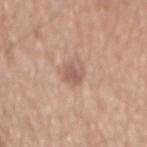follow-up — catalogued during a skin exam; not biopsied
anatomic site — the mid back
diameter — ≈2.5 mm
imaging modality — ~15 mm tile from a whole-body skin photo
subject — male, aged 68–72